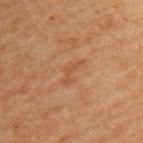follow-up: no biopsy performed (imaged during a skin exam)
subject: male, in their mid- to late 60s
location: the upper back
lesion size: about 3.5 mm
imaging modality: ~15 mm crop, total-body skin-cancer survey
lighting: cross-polarized